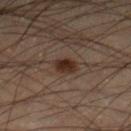Case summary:
– notes — catalogued during a skin exam; not biopsied
– lighting — cross-polarized
– image source — ~15 mm crop, total-body skin-cancer survey
– body site — the right lower leg
– automated lesion analysis — a footprint of about 5 mm², an eccentricity of roughly 0.5, and a symmetry-axis asymmetry near 0.2; a lesion color around L≈23 a*≈14 b*≈19 in CIELAB, about 9 CIELAB-L* units darker than the surrounding skin, and a normalized border contrast of about 10
– subject — male, aged around 55